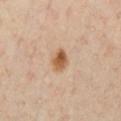Impression:
Part of a total-body skin-imaging series; this lesion was reviewed on a skin check and was not flagged for biopsy.
Clinical summary:
The tile uses cross-polarized illumination. The lesion is on the chest. A 15 mm crop from a total-body photograph taken for skin-cancer surveillance. A male patient, aged 63 to 67. Measured at roughly 3 mm in maximum diameter. The lesion-visualizer software estimated an area of roughly 4.5 mm² and a shape-asymmetry score of about 0.15 (0 = symmetric). The analysis additionally found a mean CIELAB color near L≈57 a*≈20 b*≈36, roughly 13 lightness units darker than nearby skin, and a normalized border contrast of about 9.5. It also reported an automated nevus-likeness rating near 100 out of 100 and lesion-presence confidence of about 100/100.